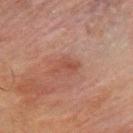Impression:
Part of a total-body skin-imaging series; this lesion was reviewed on a skin check and was not flagged for biopsy.
Image and clinical context:
A region of skin cropped from a whole-body photographic capture, roughly 15 mm wide. Imaged with cross-polarized lighting. An algorithmic analysis of the crop reported a lesion area of about 4 mm² and two-axis asymmetry of about 0.4. And it measured lesion-presence confidence of about 100/100. The lesion is located on the chest. Approximately 3 mm at its widest. The patient is a male aged 53 to 57.